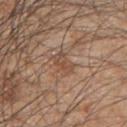This lesion was catalogued during total-body skin photography and was not selected for biopsy.
Longest diameter approximately 3.5 mm.
A male subject, in their mid-40s.
A 15 mm close-up tile from a total-body photography series done for melanoma screening.
Captured under white-light illumination.
The lesion is located on the right upper arm.